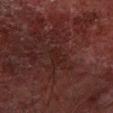Q: Was a biopsy performed?
A: imaged on a skin check; not biopsied
Q: Lesion size?
A: ≈2.5 mm
Q: Patient demographics?
A: male, roughly 70 years of age
Q: What did automated image analysis measure?
A: a lesion area of about 3.5 mm² and an eccentricity of roughly 0.8; a peripheral color-asymmetry measure near 0.5
Q: Illumination type?
A: cross-polarized illumination
Q: What kind of image is this?
A: ~15 mm crop, total-body skin-cancer survey
Q: Where on the body is the lesion?
A: the right forearm